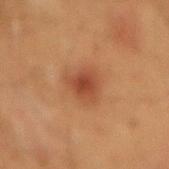Q: Was this lesion biopsied?
A: imaged on a skin check; not biopsied
Q: What is the imaging modality?
A: ~15 mm crop, total-body skin-cancer survey
Q: Patient demographics?
A: male, aged approximately 60
Q: Lesion location?
A: the abdomen